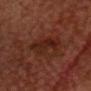workup = total-body-photography surveillance lesion; no biopsy
TBP lesion metrics = a lesion color around L≈18 a*≈19 b*≈21 in CIELAB, roughly 5 lightness units darker than nearby skin, and a normalized border contrast of about 6.5; a color-variation rating of about 2.5/10 and peripheral color asymmetry of about 1; a lesion-detection confidence of about 100/100
patient = male, in their mid- to late 60s
location = the head or neck
lesion diameter = ≈4 mm
lighting = cross-polarized illumination
image = 15 mm crop, total-body photography